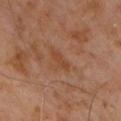workup = catalogued during a skin exam; not biopsied | location = the chest | tile lighting = cross-polarized | image source = total-body-photography crop, ~15 mm field of view | size = about 3 mm | patient = male, approximately 60 years of age.